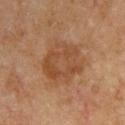{"image": {"source": "total-body photography crop", "field_of_view_mm": 15}, "site": "right upper arm", "lesion_size": {"long_diameter_mm_approx": 6.5}, "patient": {"sex": "male", "age_approx": 55}}